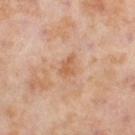notes = imaged on a skin check; not biopsied
acquisition = 15 mm crop, total-body photography
subject = female, in their mid- to late 50s
anatomic site = the right lower leg
illumination = cross-polarized illumination
lesion size = ~3 mm (longest diameter)